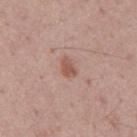Assessment: The lesion was photographed on a routine skin check and not biopsied; there is no pathology result. Context: A region of skin cropped from a whole-body photographic capture, roughly 15 mm wide. A male subject aged 43–47. The lesion is located on the back.